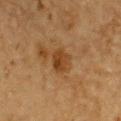{
  "biopsy_status": "not biopsied; imaged during a skin examination",
  "patient": {
    "sex": "male",
    "age_approx": "85+"
  },
  "image": {
    "source": "total-body photography crop",
    "field_of_view_mm": 15
  },
  "lighting": "cross-polarized",
  "automated_metrics": {
    "border_irregularity_0_10": 2.5,
    "color_variation_0_10": 4.0
  },
  "lesion_size": {
    "long_diameter_mm_approx": 2.5
  },
  "site": "upper back"
}